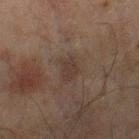Captured during whole-body skin photography for melanoma surveillance; the lesion was not biopsied. A male subject approximately 45 years of age. On the right lower leg. A region of skin cropped from a whole-body photographic capture, roughly 15 mm wide.